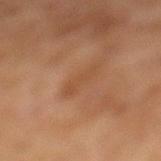Clinical impression:
Recorded during total-body skin imaging; not selected for excision or biopsy.
Background:
Captured under cross-polarized illumination. The lesion is located on the left lower leg. A female subject, roughly 60 years of age. A close-up tile cropped from a whole-body skin photograph, about 15 mm across.